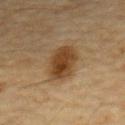Q: Was a biopsy performed?
A: imaged on a skin check; not biopsied
Q: Who is the patient?
A: male, aged 83 or over
Q: Where on the body is the lesion?
A: the chest
Q: How was this image acquired?
A: ~15 mm crop, total-body skin-cancer survey